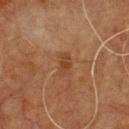  biopsy_status: not biopsied; imaged during a skin examination
  site: chest
  image:
    source: total-body photography crop
    field_of_view_mm: 15
  patient:
    sex: male
    age_approx: 75